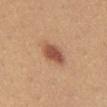Assessment:
Recorded during total-body skin imaging; not selected for excision or biopsy.
Background:
This image is a 15 mm lesion crop taken from a total-body photograph. About 3.5 mm across. Imaged with white-light lighting. A female subject, in their mid-20s. The total-body-photography lesion software estimated an automated nevus-likeness rating near 95 out of 100 and a detector confidence of about 100 out of 100 that the crop contains a lesion. The lesion is on the abdomen.